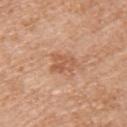biopsy status = no biopsy performed (imaged during a skin exam) | body site = the upper back | imaging modality = total-body-photography crop, ~15 mm field of view | patient = male, roughly 80 years of age.